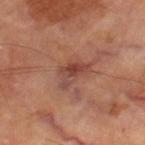The lesion was tiled from a total-body skin photograph and was not biopsied. Approximately 4 mm at its widest. A male subject aged approximately 70. Captured under cross-polarized illumination. From the left thigh. Cropped from a whole-body photographic skin survey; the tile spans about 15 mm.